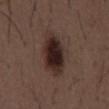  lesion_size:
    long_diameter_mm_approx: 6.5
  image:
    source: total-body photography crop
    field_of_view_mm: 15
  automated_metrics:
    area_mm2_approx: 16.0
    eccentricity: 0.85
    shape_asymmetry: 0.15
    cielab_L: 25
    cielab_a: 15
    cielab_b: 18
    vs_skin_darker_L: 13.0
    vs_skin_contrast_norm: 13.0
    border_irregularity_0_10: 2.0
  patient:
    sex: male
    age_approx: 50
  lighting: white-light
  site: abdomen